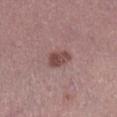Imaged during a routine full-body skin examination; the lesion was not biopsied and no histopathology is available.
The tile uses white-light illumination.
Cropped from a whole-body photographic skin survey; the tile spans about 15 mm.
Measured at roughly 3.5 mm in maximum diameter.
A male subject, aged 43–47.
The lesion is on the right lower leg.